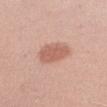This lesion was catalogued during total-body skin photography and was not selected for biopsy. The lesion-visualizer software estimated an area of roughly 8.5 mm², an eccentricity of roughly 0.65, and a symmetry-axis asymmetry near 0.2. The software also gave border irregularity of about 2 on a 0–10 scale, a within-lesion color-variation index near 2/10, and peripheral color asymmetry of about 0.5. On the right upper arm. A female patient, aged 28 to 32. A 15 mm close-up tile from a total-body photography series done for melanoma screening. Captured under white-light illumination.